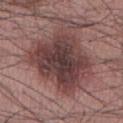follow-up: total-body-photography surveillance lesion; no biopsy
patient: male, aged 53 to 57
anatomic site: the abdomen
lesion diameter: ≈7 mm
acquisition: ~15 mm tile from a whole-body skin photo